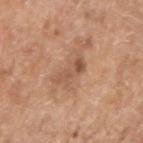Imaged during a routine full-body skin examination; the lesion was not biopsied and no histopathology is available. A lesion tile, about 15 mm wide, cut from a 3D total-body photograph. The recorded lesion diameter is about 4 mm. An algorithmic analysis of the crop reported a lesion area of about 5.5 mm², a shape eccentricity near 0.9, and a shape-asymmetry score of about 0.35 (0 = symmetric). The analysis additionally found a classifier nevus-likeness of about 0/100 and a lesion-detection confidence of about 100/100. Imaged with white-light lighting. From the right upper arm. A male subject aged 58 to 62.